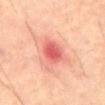{
  "biopsy_status": "not biopsied; imaged during a skin examination",
  "lighting": "cross-polarized",
  "image": {
    "source": "total-body photography crop",
    "field_of_view_mm": 15
  },
  "lesion_size": {
    "long_diameter_mm_approx": 3.5
  },
  "site": "abdomen",
  "patient": {
    "sex": "male",
    "age_approx": 60
  }
}